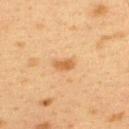workup = no biopsy performed (imaged during a skin exam).